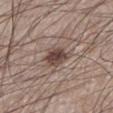biopsy status: catalogued during a skin exam; not biopsied | site: the left lower leg | patient: male, roughly 60 years of age | image: ~15 mm tile from a whole-body skin photo.